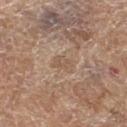Clinical impression: Part of a total-body skin-imaging series; this lesion was reviewed on a skin check and was not flagged for biopsy. Clinical summary: The lesion is on the left thigh. A female patient aged 73 to 77. Approximately 2.5 mm at its widest. A 15 mm close-up extracted from a 3D total-body photography capture. Captured under white-light illumination.